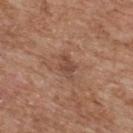Part of a total-body skin-imaging series; this lesion was reviewed on a skin check and was not flagged for biopsy.
A roughly 15 mm field-of-view crop from a total-body skin photograph.
The tile uses white-light illumination.
The subject is a male aged 63–67.
About 3 mm across.
Located on the upper back.
The total-body-photography lesion software estimated an area of roughly 4.5 mm², a shape eccentricity near 0.7, and two-axis asymmetry of about 0.25. And it measured a border-irregularity index near 3/10 and radial color variation of about 0.5. It also reported an automated nevus-likeness rating near 0 out of 100 and a detector confidence of about 100 out of 100 that the crop contains a lesion.